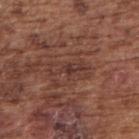Clinical impression: No biopsy was performed on this lesion — it was imaged during a full skin examination and was not determined to be concerning. Context: A male subject, in their mid- to late 70s. Automated image analysis of the tile measured a mean CIELAB color near L≈37 a*≈21 b*≈24 and a normalized lesion–skin contrast near 5.5. Located on the arm. A close-up tile cropped from a whole-body skin photograph, about 15 mm across. Captured under white-light illumination.